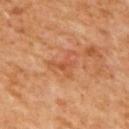– notes · no biopsy performed (imaged during a skin exam)
– subject · male, aged approximately 65
– anatomic site · the mid back
– lesion diameter · about 3.5 mm
– tile lighting · cross-polarized illumination
– automated metrics · a lesion color around L≈51 a*≈26 b*≈37 in CIELAB, roughly 7 lightness units darker than nearby skin, and a normalized border contrast of about 5; a border-irregularity rating of about 8/10, a within-lesion color-variation index near 2/10, and peripheral color asymmetry of about 0.5
– imaging modality · 15 mm crop, total-body photography